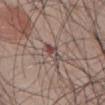Case summary:
- biopsy status: catalogued during a skin exam; not biopsied
- diameter: ~2.5 mm (longest diameter)
- site: the abdomen
- illumination: white-light
- patient: male, aged approximately 50
- image source: 15 mm crop, total-body photography
- automated metrics: a lesion area of about 3.5 mm² and an eccentricity of roughly 0.85; a mean CIELAB color near L≈46 a*≈18 b*≈20, roughly 10 lightness units darker than nearby skin, and a normalized lesion–skin contrast near 7.5; a nevus-likeness score of about 0/100 and a detector confidence of about 100 out of 100 that the crop contains a lesion